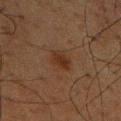{"biopsy_status": "not biopsied; imaged during a skin examination", "site": "chest", "patient": {"sex": "male", "age_approx": 60}, "automated_metrics": {"area_mm2_approx": 5.0, "eccentricity": 0.75, "cielab_L": 24, "cielab_a": 16, "cielab_b": 23, "vs_skin_darker_L": 6.0, "vs_skin_contrast_norm": 7.0}, "lesion_size": {"long_diameter_mm_approx": 3.0}, "image": {"source": "total-body photography crop", "field_of_view_mm": 15}}